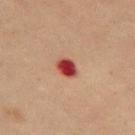Assessment: No biopsy was performed on this lesion — it was imaged during a full skin examination and was not determined to be concerning. Background: The recorded lesion diameter is about 2.5 mm. A female patient about 70 years old. The tile uses cross-polarized illumination. A 15 mm close-up tile from a total-body photography series done for melanoma screening. Located on the left thigh.